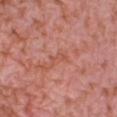Acquisition and patient details:
A female patient, approximately 40 years of age. Cropped from a total-body skin-imaging series; the visible field is about 15 mm. On the right lower leg.